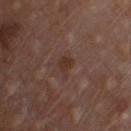{
  "biopsy_status": "not biopsied; imaged during a skin examination",
  "site": "front of the torso",
  "lighting": "white-light",
  "image": {
    "source": "total-body photography crop",
    "field_of_view_mm": 15
  },
  "lesion_size": {
    "long_diameter_mm_approx": 2.5
  },
  "patient": {
    "sex": "male",
    "age_approx": 80
  }
}